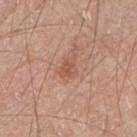Captured during whole-body skin photography for melanoma surveillance; the lesion was not biopsied.
A male patient roughly 65 years of age.
Cropped from a total-body skin-imaging series; the visible field is about 15 mm.
The lesion is on the right forearm.
The total-body-photography lesion software estimated a footprint of about 3.5 mm² and a shape eccentricity near 0.7. It also reported a mean CIELAB color near L≈54 a*≈23 b*≈30, a lesion–skin lightness drop of about 8, and a normalized border contrast of about 5.5. The software also gave border irregularity of about 3.5 on a 0–10 scale, a within-lesion color-variation index near 1.5/10, and peripheral color asymmetry of about 0.5. It also reported a nevus-likeness score of about 5/100 and lesion-presence confidence of about 100/100.
Longest diameter approximately 2.5 mm.
The tile uses white-light illumination.